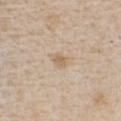Impression: The lesion was tiled from a total-body skin photograph and was not biopsied. Clinical summary: Imaged with white-light lighting. A female subject, about 45 years old. Automated image analysis of the tile measured a lesion area of about 3.5 mm², an outline eccentricity of about 0.7 (0 = round, 1 = elongated), and a symmetry-axis asymmetry near 0.3. The software also gave a nevus-likeness score of about 5/100 and lesion-presence confidence of about 100/100. A close-up tile cropped from a whole-body skin photograph, about 15 mm across. From the chest.